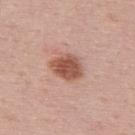Q: What is the anatomic site?
A: the upper back
Q: How was this image acquired?
A: 15 mm crop, total-body photography
Q: What lighting was used for the tile?
A: white-light illumination
Q: What is the lesion's diameter?
A: about 4 mm
Q: Patient demographics?
A: male, aged 48–52